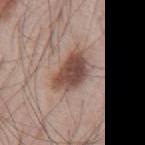biopsy status: total-body-photography surveillance lesion; no biopsy
imaging modality: ~15 mm crop, total-body skin-cancer survey
site: the mid back
illumination: white-light illumination
patient: male, aged 43 to 47
size: ~5 mm (longest diameter)